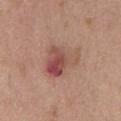Imaged during a routine full-body skin examination; the lesion was not biopsied and no histopathology is available.
A male patient aged approximately 55.
A 15 mm crop from a total-body photograph taken for skin-cancer surveillance.
This is a white-light tile.
Automated tile analysis of the lesion measured an average lesion color of about L≈51 a*≈23 b*≈25 (CIELAB), a lesion–skin lightness drop of about 10, and a lesion-to-skin contrast of about 7.5 (normalized; higher = more distinct). The software also gave an automated nevus-likeness rating near 0 out of 100.
The recorded lesion diameter is about 5.5 mm.
From the chest.